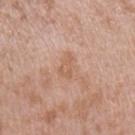{"biopsy_status": "not biopsied; imaged during a skin examination", "lesion_size": {"long_diameter_mm_approx": 3.0}, "lighting": "white-light", "site": "left upper arm", "patient": {"sex": "male", "age_approx": 40}, "image": {"source": "total-body photography crop", "field_of_view_mm": 15}}